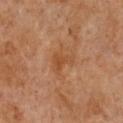Imaged during a routine full-body skin examination; the lesion was not biopsied and no histopathology is available. The patient is a female aged around 50. Approximately 3 mm at its widest. A 15 mm close-up extracted from a 3D total-body photography capture. This is a cross-polarized tile. From the chest.